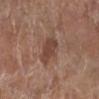<case>
<biopsy_status>not biopsied; imaged during a skin examination</biopsy_status>
<patient>
  <sex>female</sex>
  <age_approx>80</age_approx>
</patient>
<site>right lower leg</site>
<image>
  <source>total-body photography crop</source>
  <field_of_view_mm>15</field_of_view_mm>
</image>
<lesion_size>
  <long_diameter_mm_approx>4.0</long_diameter_mm_approx>
</lesion_size>
<lighting>white-light</lighting>
</case>